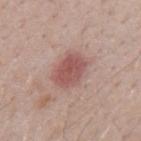The lesion was photographed on a routine skin check and not biopsied; there is no pathology result. From the mid back. About 4 mm across. Cropped from a total-body skin-imaging series; the visible field is about 15 mm. Imaged with white-light lighting. The subject is a male aged 38–42.